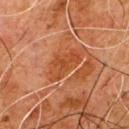Clinical impression: Imaged during a routine full-body skin examination; the lesion was not biopsied and no histopathology is available. Context: The lesion is on the chest. A 15 mm close-up tile from a total-body photography series done for melanoma screening. The patient is a male in their 80s. The lesion-visualizer software estimated a lesion color around L≈37 a*≈24 b*≈33 in CIELAB and about 6 CIELAB-L* units darker than the surrounding skin. The analysis additionally found a classifier nevus-likeness of about 0/100 and a lesion-detection confidence of about 90/100. Captured under cross-polarized illumination.